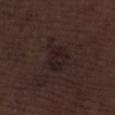Imaged during a routine full-body skin examination; the lesion was not biopsied and no histopathology is available.
The patient is a male in their 70s.
The lesion-visualizer software estimated a lesion area of about 10 mm², an outline eccentricity of about 0.75 (0 = round, 1 = elongated), and two-axis asymmetry of about 0.35. And it measured about 5 CIELAB-L* units darker than the surrounding skin.
The lesion is located on the lower back.
The tile uses white-light illumination.
The recorded lesion diameter is about 5 mm.
This image is a 15 mm lesion crop taken from a total-body photograph.